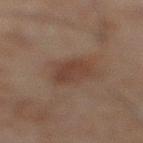Q: Who is the patient?
A: male, aged around 45
Q: How large is the lesion?
A: about 4.5 mm
Q: Automated lesion metrics?
A: about 6 CIELAB-L* units darker than the surrounding skin and a normalized border contrast of about 6
Q: How was this image acquired?
A: ~15 mm crop, total-body skin-cancer survey
Q: Lesion location?
A: the right lower leg